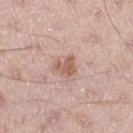Clinical impression:
Captured during whole-body skin photography for melanoma surveillance; the lesion was not biopsied.
Acquisition and patient details:
A roughly 15 mm field-of-view crop from a total-body skin photograph. The recorded lesion diameter is about 3 mm. Captured under white-light illumination. The lesion is located on the left thigh. A male patient aged 48 to 52. The lesion-visualizer software estimated a lesion color around L≈59 a*≈21 b*≈27 in CIELAB and a normalized lesion–skin contrast near 7. And it measured border irregularity of about 3.5 on a 0–10 scale, a color-variation rating of about 2.5/10, and peripheral color asymmetry of about 1. The software also gave an automated nevus-likeness rating near 15 out of 100 and a lesion-detection confidence of about 100/100.